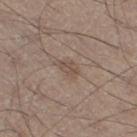Clinical impression: Imaged during a routine full-body skin examination; the lesion was not biopsied and no histopathology is available. Background: This is a white-light tile. Approximately 2.5 mm at its widest. The patient is a male aged around 60. A region of skin cropped from a whole-body photographic capture, roughly 15 mm wide. The lesion is located on the right thigh. Automated image analysis of the tile measured a lesion–skin lightness drop of about 7.